The lesion is on the chest; a male patient aged around 50; a 15 mm close-up tile from a total-body photography series done for melanoma screening.
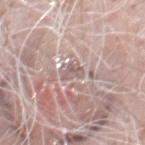This is a white-light tile. Histopathologically confirmed as a pigmented benign keratosis, classified as a benign lesion.